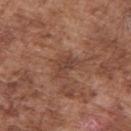A male patient aged 73 to 77. On the right upper arm. Imaged with white-light lighting. A roughly 15 mm field-of-view crop from a total-body skin photograph. The lesion-visualizer software estimated a shape eccentricity near 0.7. About 3.5 mm across.